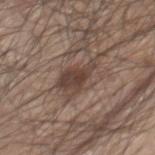Impression:
Imaged during a routine full-body skin examination; the lesion was not biopsied and no histopathology is available.
Image and clinical context:
A male patient roughly 80 years of age. A 15 mm crop from a total-body photograph taken for skin-cancer surveillance. The lesion is located on the chest. Longest diameter approximately 6 mm. An algorithmic analysis of the crop reported an outline eccentricity of about 0.9 (0 = round, 1 = elongated) and two-axis asymmetry of about 0.5. The software also gave an average lesion color of about L≈42 a*≈15 b*≈22 (CIELAB), a lesion–skin lightness drop of about 10, and a normalized border contrast of about 8. The analysis additionally found a classifier nevus-likeness of about 35/100.